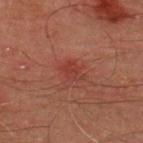The lesion was photographed on a routine skin check and not biopsied; there is no pathology result.
The lesion is located on the upper back.
The total-body-photography lesion software estimated an average lesion color of about L≈32 a*≈25 b*≈24 (CIELAB), roughly 5 lightness units darker than nearby skin, and a normalized lesion–skin contrast near 5.5. It also reported a border-irregularity rating of about 2.5/10, a color-variation rating of about 2/10, and peripheral color asymmetry of about 1.
A male patient in their mid-40s.
A close-up tile cropped from a whole-body skin photograph, about 15 mm across.
About 2.5 mm across.